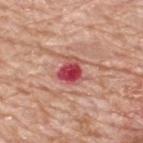<case>
  <lesion_size>
    <long_diameter_mm_approx>3.0</long_diameter_mm_approx>
  </lesion_size>
  <image>
    <source>total-body photography crop</source>
    <field_of_view_mm>15</field_of_view_mm>
  </image>
  <lighting>white-light</lighting>
  <patient>
    <sex>male</sex>
    <age_approx>80</age_approx>
  </patient>
  <site>upper back</site>
</case>